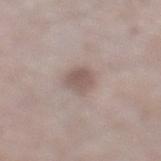Findings:
* workup — total-body-photography surveillance lesion; no biopsy
* automated metrics — an area of roughly 5.5 mm², an eccentricity of roughly 0.65, and a symmetry-axis asymmetry near 0.25; a border-irregularity index near 2/10, a color-variation rating of about 2.5/10, and radial color variation of about 1; a classifier nevus-likeness of about 75/100 and a lesion-detection confidence of about 100/100
* patient — male, aged approximately 65
* tile lighting — white-light illumination
* lesion size — about 3 mm
* image source — 15 mm crop, total-body photography
* body site — the left lower leg